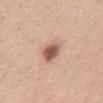Clinical impression:
Imaged during a routine full-body skin examination; the lesion was not biopsied and no histopathology is available.
Clinical summary:
Located on the abdomen. A female patient in their mid- to late 50s. The tile uses white-light illumination. The lesion's longest dimension is about 3 mm. This image is a 15 mm lesion crop taken from a total-body photograph.